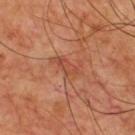Case summary:
– workup · catalogued during a skin exam; not biopsied
– body site · the chest
– illumination · cross-polarized illumination
– image source · 15 mm crop, total-body photography
– subject · male, aged around 65
– size · ~4 mm (longest diameter)
– automated metrics · an average lesion color of about L≈46 a*≈26 b*≈32 (CIELAB), about 7 CIELAB-L* units darker than the surrounding skin, and a normalized border contrast of about 5; a border-irregularity rating of about 6/10 and a color-variation rating of about 3.5/10; a nevus-likeness score of about 0/100 and a lesion-detection confidence of about 100/100